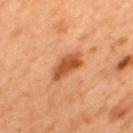Q: Is there a histopathology result?
A: total-body-photography surveillance lesion; no biopsy
Q: What is the lesion's diameter?
A: ≈4.5 mm
Q: Illumination type?
A: cross-polarized illumination
Q: Lesion location?
A: the back
Q: What are the patient's age and sex?
A: male, roughly 50 years of age
Q: Automated lesion metrics?
A: an eccentricity of roughly 0.9; an average lesion color of about L≈54 a*≈29 b*≈42 (CIELAB), a lesion–skin lightness drop of about 13, and a lesion-to-skin contrast of about 9 (normalized; higher = more distinct); a border-irregularity index near 2.5/10, a within-lesion color-variation index near 3/10, and a peripheral color-asymmetry measure near 1
Q: What kind of image is this?
A: ~15 mm crop, total-body skin-cancer survey